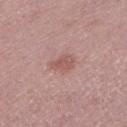  biopsy_status: not biopsied; imaged during a skin examination
  lesion_size:
    long_diameter_mm_approx: 2.5
  patient:
    sex: male
    age_approx: 75
  image:
    source: total-body photography crop
    field_of_view_mm: 15
  lighting: white-light
  site: left lower leg